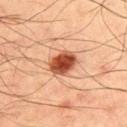workup: total-body-photography surveillance lesion; no biopsy | location: the upper back | illumination: cross-polarized illumination | subject: male, approximately 50 years of age | lesion size: ~3.5 mm (longest diameter) | image: ~15 mm tile from a whole-body skin photo.